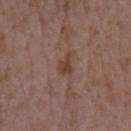Imaged during a routine full-body skin examination; the lesion was not biopsied and no histopathology is available. Located on the left forearm. The total-body-photography lesion software estimated a mean CIELAB color near L≈41 a*≈19 b*≈25, roughly 8 lightness units darker than nearby skin, and a normalized border contrast of about 7. About 2.5 mm across. Cropped from a whole-body photographic skin survey; the tile spans about 15 mm. The tile uses white-light illumination. The subject is a female in their mid-30s.